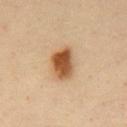{
  "biopsy_status": "not biopsied; imaged during a skin examination",
  "lighting": "cross-polarized",
  "patient": {
    "sex": "male",
    "age_approx": 35
  },
  "lesion_size": {
    "long_diameter_mm_approx": 4.0
  },
  "image": {
    "source": "total-body photography crop",
    "field_of_view_mm": 15
  },
  "site": "front of the torso"
}